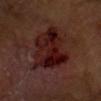Impression: Imaged during a routine full-body skin examination; the lesion was not biopsied and no histopathology is available. Clinical summary: The subject is a male about 70 years old. The lesion is located on the front of the torso. Cropped from a whole-body photographic skin survey; the tile spans about 15 mm. Automated image analysis of the tile measured an area of roughly 26 mm², an eccentricity of roughly 0.7, and two-axis asymmetry of about 0.3. The software also gave a mean CIELAB color near L≈19 a*≈23 b*≈20, about 9 CIELAB-L* units darker than the surrounding skin, and a normalized border contrast of about 10.5. The software also gave a border-irregularity index near 4/10, a color-variation rating of about 7.5/10, and radial color variation of about 2.5. It also reported an automated nevus-likeness rating near 0 out of 100.